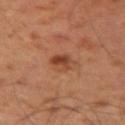{"biopsy_status": "not biopsied; imaged during a skin examination", "automated_metrics": {"area_mm2_approx": 6.0, "eccentricity": 0.65, "shape_asymmetry": 0.15, "nevus_likeness_0_100": 70, "lesion_detection_confidence_0_100": 100}, "site": "left upper arm", "patient": {"sex": "male", "age_approx": 50}, "lighting": "cross-polarized", "lesion_size": {"long_diameter_mm_approx": 3.0}, "image": {"source": "total-body photography crop", "field_of_view_mm": 15}}